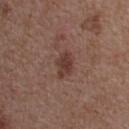Recorded during total-body skin imaging; not selected for excision or biopsy. The lesion is located on the head or neck. A female patient aged around 40. Captured under white-light illumination. Cropped from a whole-body photographic skin survey; the tile spans about 15 mm.